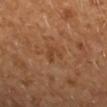Recorded during total-body skin imaging; not selected for excision or biopsy. Cropped from a whole-body photographic skin survey; the tile spans about 15 mm. On the left forearm. This is a cross-polarized tile. Automated image analysis of the tile measured a border-irregularity index near 4.5/10, internal color variation of about 2 on a 0–10 scale, and radial color variation of about 1. The lesion's longest dimension is about 2.5 mm. A female patient aged around 65.